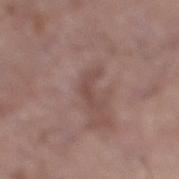notes: total-body-photography surveillance lesion; no biopsy
anatomic site: the right lower leg
acquisition: total-body-photography crop, ~15 mm field of view
lesion diameter: ~3 mm (longest diameter)
patient: male, aged approximately 70
illumination: white-light illumination
TBP lesion metrics: an area of roughly 2.5 mm², an outline eccentricity of about 0.9 (0 = round, 1 = elongated), and a shape-asymmetry score of about 0.6 (0 = symmetric); a lesion color around L≈46 a*≈19 b*≈21 in CIELAB, about 7 CIELAB-L* units darker than the surrounding skin, and a normalized border contrast of about 5.5; a border-irregularity rating of about 7.5/10, a within-lesion color-variation index near 0/10, and a peripheral color-asymmetry measure near 0; a classifier nevus-likeness of about 0/100 and a lesion-detection confidence of about 70/100